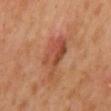biopsy status: total-body-photography surveillance lesion; no biopsy
diameter: about 5.5 mm
anatomic site: the abdomen
subject: male, aged 43 to 47
imaging modality: total-body-photography crop, ~15 mm field of view
automated lesion analysis: a mean CIELAB color near L≈47 a*≈26 b*≈32, about 9 CIELAB-L* units darker than the surrounding skin, and a normalized lesion–skin contrast near 6.5; a border-irregularity rating of about 4.5/10, a within-lesion color-variation index near 6/10, and peripheral color asymmetry of about 2; a classifier nevus-likeness of about 10/100 and a detector confidence of about 100 out of 100 that the crop contains a lesion